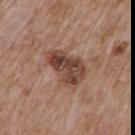No biopsy was performed on this lesion — it was imaged during a full skin examination and was not determined to be concerning.
A male patient, aged approximately 60.
Located on the back.
A region of skin cropped from a whole-body photographic capture, roughly 15 mm wide.